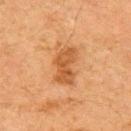The tile uses cross-polarized illumination. A 15 mm close-up extracted from a 3D total-body photography capture. The patient is a male in their mid- to late 60s. The total-body-photography lesion software estimated a border-irregularity rating of about 3.5/10, a color-variation rating of about 3/10, and peripheral color asymmetry of about 1. It also reported an automated nevus-likeness rating near 55 out of 100. Located on the right upper arm.